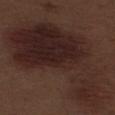workup: no biopsy performed (imaged during a skin exam); imaging modality: 15 mm crop, total-body photography; subject: male, roughly 70 years of age; anatomic site: the left thigh.